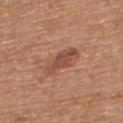<case>
<biopsy_status>not biopsied; imaged during a skin examination</biopsy_status>
<site>back</site>
<automated_metrics>
  <area_mm2_approx>8.0</area_mm2_approx>
  <eccentricity>0.9</eccentricity>
  <shape_asymmetry>0.2</shape_asymmetry>
  <vs_skin_darker_L>10.0</vs_skin_darker_L>
  <vs_skin_contrast_norm>7.0</vs_skin_contrast_norm>
  <border_irregularity_0_10>3.0</border_irregularity_0_10>
  <color_variation_0_10>3.0</color_variation_0_10>
  <peripheral_color_asymmetry>1.0</peripheral_color_asymmetry>
  <nevus_likeness_0_100>55</nevus_likeness_0_100>
  <lesion_detection_confidence_0_100>100</lesion_detection_confidence_0_100>
</automated_metrics>
<image>
  <source>total-body photography crop</source>
  <field_of_view_mm>15</field_of_view_mm>
</image>
<lighting>white-light</lighting>
<patient>
  <sex>male</sex>
  <age_approx>70</age_approx>
</patient>
</case>